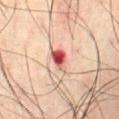| field | value |
|---|---|
| workup | catalogued during a skin exam; not biopsied |
| image source | total-body-photography crop, ~15 mm field of view |
| subject | male, approximately 70 years of age |
| size | about 3 mm |
| automated lesion analysis | a footprint of about 5 mm², an outline eccentricity of about 0.75 (0 = round, 1 = elongated), and a shape-asymmetry score of about 0.25 (0 = symmetric); a border-irregularity rating of about 2/10, a within-lesion color-variation index near 10/10, and peripheral color asymmetry of about 3.5; an automated nevus-likeness rating near 0 out of 100 |
| tile lighting | cross-polarized |
| anatomic site | the abdomen |